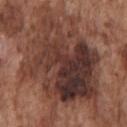Q: Is there a histopathology result?
A: no biopsy performed (imaged during a skin exam)
Q: How was the tile lit?
A: white-light illumination
Q: Who is the patient?
A: male, aged 73–77
Q: Lesion size?
A: ~12 mm (longest diameter)
Q: Automated lesion metrics?
A: a footprint of about 65 mm², an outline eccentricity of about 0.7 (0 = round, 1 = elongated), and a symmetry-axis asymmetry near 0.25; a mean CIELAB color near L≈36 a*≈20 b*≈23 and roughly 12 lightness units darker than nearby skin
Q: What kind of image is this?
A: total-body-photography crop, ~15 mm field of view
Q: Where on the body is the lesion?
A: the chest